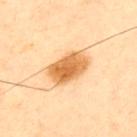Impression: Recorded during total-body skin imaging; not selected for excision or biopsy. Image and clinical context: A male patient aged 43 to 47. The lesion is on the upper back. Cropped from a whole-body photographic skin survey; the tile spans about 15 mm.